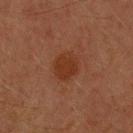Findings:
• biopsy status — no biopsy performed (imaged during a skin exam)
• size — ~3.5 mm (longest diameter)
• imaging modality — ~15 mm tile from a whole-body skin photo
• patient — male, aged 68 to 72
• site — the right upper arm